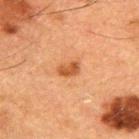biopsy status=total-body-photography surveillance lesion; no biopsy
subject=male, aged approximately 50
imaging modality=~15 mm crop, total-body skin-cancer survey
anatomic site=the upper back
lesion size=about 2.5 mm
tile lighting=cross-polarized